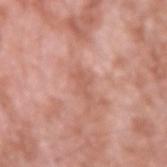Q: Was a biopsy performed?
A: imaged on a skin check; not biopsied